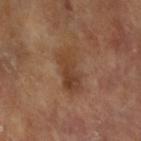A female patient aged around 75.
A 15 mm close-up tile from a total-body photography series done for melanoma screening.
From the left forearm.